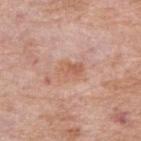Clinical summary: An algorithmic analysis of the crop reported an area of roughly 5.5 mm² and an outline eccentricity of about 0.7 (0 = round, 1 = elongated). A female patient, aged 68 to 72. A close-up tile cropped from a whole-body skin photograph, about 15 mm across. The recorded lesion diameter is about 3 mm. From the right forearm.